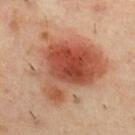Case summary:
• automated lesion analysis: a lesion–skin lightness drop of about 14 and a lesion-to-skin contrast of about 9.5 (normalized; higher = more distinct); a nevus-likeness score of about 100/100 and a detector confidence of about 100 out of 100 that the crop contains a lesion
• lesion diameter: about 8.5 mm
• anatomic site: the upper back
• subject: male, aged around 40
• lighting: cross-polarized
• image source: ~15 mm tile from a whole-body skin photo